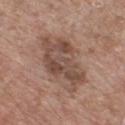  biopsy_status: not biopsied; imaged during a skin examination
  patient:
    sex: male
    age_approx: 55
  image:
    source: total-body photography crop
    field_of_view_mm: 15
  site: chest
  automated_metrics:
    area_mm2_approx: 25.0
    eccentricity: 0.85
    shape_asymmetry: 0.25
    cielab_L: 48
    cielab_a: 18
    cielab_b: 25
    vs_skin_contrast_norm: 7.5